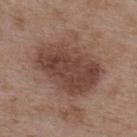No biopsy was performed on this lesion — it was imaged during a full skin examination and was not determined to be concerning.
An algorithmic analysis of the crop reported a border-irregularity index near 2/10 and peripheral color asymmetry of about 1.5.
The lesion is on the back.
This is a white-light tile.
The subject is a male approximately 50 years of age.
Approximately 8 mm at its widest.
A 15 mm crop from a total-body photograph taken for skin-cancer surveillance.